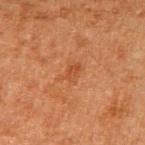Q: Was a biopsy performed?
A: total-body-photography surveillance lesion; no biopsy
Q: What is the imaging modality?
A: ~15 mm crop, total-body skin-cancer survey
Q: Illumination type?
A: cross-polarized
Q: What are the patient's age and sex?
A: male, in their mid-60s
Q: What is the anatomic site?
A: the right upper arm
Q: What did automated image analysis measure?
A: a shape eccentricity near 0.75 and a shape-asymmetry score of about 0.3 (0 = symmetric); an average lesion color of about L≈43 a*≈25 b*≈36 (CIELAB)